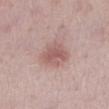Assessment: No biopsy was performed on this lesion — it was imaged during a full skin examination and was not determined to be concerning. Background: Cropped from a total-body skin-imaging series; the visible field is about 15 mm. On the left lower leg. Measured at roughly 3.5 mm in maximum diameter. This is a white-light tile. A female patient aged approximately 40. An algorithmic analysis of the crop reported a lesion area of about 9 mm², an eccentricity of roughly 0.5, and a shape-asymmetry score of about 0.25 (0 = symmetric). The software also gave a border-irregularity index near 2.5/10 and radial color variation of about 1.